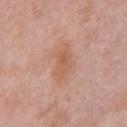Recorded during total-body skin imaging; not selected for excision or biopsy. Approximately 4.5 mm at its widest. The lesion is on the chest. A male subject, approximately 55 years of age. A roughly 15 mm field-of-view crop from a total-body skin photograph. Automated image analysis of the tile measured a footprint of about 7 mm². It also reported a lesion color around L≈58 a*≈22 b*≈30 in CIELAB, a lesion–skin lightness drop of about 7, and a normalized border contrast of about 6. And it measured a within-lesion color-variation index near 3/10 and a peripheral color-asymmetry measure near 1. Imaged with white-light lighting.